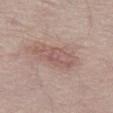Automated tile analysis of the lesion measured a lesion area of about 11 mm², an outline eccentricity of about 0.9 (0 = round, 1 = elongated), and two-axis asymmetry of about 0.3.
The patient is a male roughly 75 years of age.
Captured under white-light illumination.
Cropped from a whole-body photographic skin survey; the tile spans about 15 mm.
The lesion is on the left thigh.